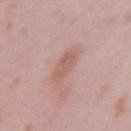Assessment:
No biopsy was performed on this lesion — it was imaged during a full skin examination and was not determined to be concerning.
Clinical summary:
The subject is a female aged around 15. A lesion tile, about 15 mm wide, cut from a 3D total-body photograph. Located on the left upper arm. About 3.5 mm across. Imaged with white-light lighting.